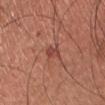This lesion was catalogued during total-body skin photography and was not selected for biopsy. A male patient approximately 35 years of age. A 15 mm close-up extracted from a 3D total-body photography capture. From the chest.